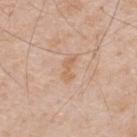The lesion was photographed on a routine skin check and not biopsied; there is no pathology result. A 15 mm close-up extracted from a 3D total-body photography capture. The subject is a male in their 50s. About 3 mm across. The lesion is located on the upper back. The lesion-visualizer software estimated a nevus-likeness score of about 0/100. The tile uses white-light illumination.